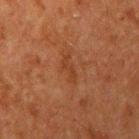biopsy status = total-body-photography surveillance lesion; no biopsy | location = the right upper arm | size = ~3 mm (longest diameter) | subject = male, aged 58 to 62 | image = ~15 mm tile from a whole-body skin photo | TBP lesion metrics = a footprint of about 3.5 mm² and a symmetry-axis asymmetry near 0.4; an average lesion color of about L≈31 a*≈21 b*≈28 (CIELAB), a lesion–skin lightness drop of about 5, and a normalized lesion–skin contrast near 5; border irregularity of about 5 on a 0–10 scale, a color-variation rating of about 0/10, and a peripheral color-asymmetry measure near 0; a classifier nevus-likeness of about 0/100 and lesion-presence confidence of about 100/100.